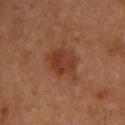notes — total-body-photography surveillance lesion; no biopsy | automated metrics — a mean CIELAB color near L≈37 a*≈23 b*≈31, a lesion–skin lightness drop of about 8, and a normalized border contrast of about 7; a detector confidence of about 100 out of 100 that the crop contains a lesion | image — 15 mm crop, total-body photography | subject — male, aged around 55 | lighting — cross-polarized illumination | body site — the upper back.